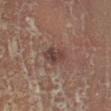Impression: No biopsy was performed on this lesion — it was imaged during a full skin examination and was not determined to be concerning. Background: A female subject aged 53 to 57. The lesion's longest dimension is about 3 mm. Cropped from a whole-body photographic skin survey; the tile spans about 15 mm. Automated image analysis of the tile measured a lesion area of about 6 mm², a shape eccentricity near 0.75, and a shape-asymmetry score of about 0.15 (0 = symmetric). The analysis additionally found a within-lesion color-variation index near 3.5/10 and peripheral color asymmetry of about 1. Captured under cross-polarized illumination. The lesion is located on the right lower leg.